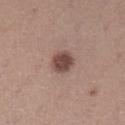Imaged during a routine full-body skin examination; the lesion was not biopsied and no histopathology is available.